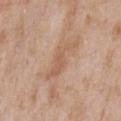The lesion was tiled from a total-body skin photograph and was not biopsied. The lesion is on the chest. Captured under white-light illumination. An algorithmic analysis of the crop reported an outline eccentricity of about 0.95 (0 = round, 1 = elongated). The analysis additionally found a mean CIELAB color near L≈58 a*≈19 b*≈31, about 8 CIELAB-L* units darker than the surrounding skin, and a lesion-to-skin contrast of about 5.5 (normalized; higher = more distinct). And it measured border irregularity of about 7.5 on a 0–10 scale, internal color variation of about 1 on a 0–10 scale, and peripheral color asymmetry of about 0. It also reported a classifier nevus-likeness of about 0/100 and lesion-presence confidence of about 90/100. Cropped from a whole-body photographic skin survey; the tile spans about 15 mm. The subject is a male approximately 65 years of age. The recorded lesion diameter is about 6 mm.